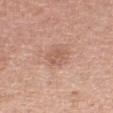{"biopsy_status": "not biopsied; imaged during a skin examination", "site": "right thigh", "lighting": "white-light", "image": {"source": "total-body photography crop", "field_of_view_mm": 15}, "patient": {"sex": "female", "age_approx": 55}}